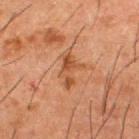Part of a total-body skin-imaging series; this lesion was reviewed on a skin check and was not flagged for biopsy. The recorded lesion diameter is about 4 mm. Captured under cross-polarized illumination. A roughly 15 mm field-of-view crop from a total-body skin photograph. The total-body-photography lesion software estimated a normalized lesion–skin contrast near 7. And it measured a border-irregularity index near 7/10, a color-variation rating of about 1.5/10, and a peripheral color-asymmetry measure near 0.5. The lesion is on the upper back. The subject is a male aged approximately 50.